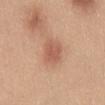<tbp_lesion>
<biopsy_status>not biopsied; imaged during a skin examination</biopsy_status>
<lesion_size>
  <long_diameter_mm_approx>3.0</long_diameter_mm_approx>
</lesion_size>
<site>chest</site>
<image>
  <source>total-body photography crop</source>
  <field_of_view_mm>15</field_of_view_mm>
</image>
<patient>
  <sex>female</sex>
  <age_approx>30</age_approx>
</patient>
<automated_metrics>
  <area_mm2_approx>6.5</area_mm2_approx>
  <shape_asymmetry>0.15</shape_asymmetry>
</automated_metrics>
</tbp_lesion>